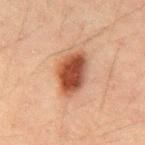{
  "automated_metrics": {
    "cielab_L": 39,
    "cielab_a": 22,
    "cielab_b": 28,
    "vs_skin_contrast_norm": 11.5,
    "color_variation_0_10": 5.5,
    "peripheral_color_asymmetry": 1.5,
    "nevus_likeness_0_100": 100,
    "lesion_detection_confidence_0_100": 100
  },
  "lesion_size": {
    "long_diameter_mm_approx": 5.0
  },
  "patient": {
    "sex": "male",
    "age_approx": 50
  },
  "site": "mid back",
  "lighting": "cross-polarized",
  "image": {
    "source": "total-body photography crop",
    "field_of_view_mm": 15
  }
}